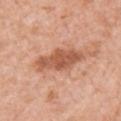Assessment:
Recorded during total-body skin imaging; not selected for excision or biopsy.
Background:
Measured at roughly 6 mm in maximum diameter. This is a white-light tile. From the right upper arm. A female patient aged approximately 40. A 15 mm crop from a total-body photograph taken for skin-cancer surveillance.